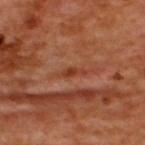Findings:
– follow-up: catalogued during a skin exam; not biopsied
– patient: male, aged around 50
– lesion size: ≈2.5 mm
– automated metrics: a lesion color around L≈39 a*≈29 b*≈33 in CIELAB, roughly 8 lightness units darker than nearby skin, and a lesion-to-skin contrast of about 7.5 (normalized; higher = more distinct); a border-irregularity index near 5/10, internal color variation of about 0 on a 0–10 scale, and radial color variation of about 0
– body site: the upper back
– illumination: cross-polarized illumination
– imaging modality: ~15 mm crop, total-body skin-cancer survey